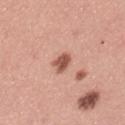The lesion was tiled from a total-body skin photograph and was not biopsied.
A female patient, aged 33 to 37.
From the left thigh.
A 15 mm crop from a total-body photograph taken for skin-cancer surveillance.